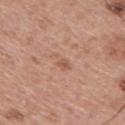Case summary:
* TBP lesion metrics — a footprint of about 3.5 mm², a shape eccentricity near 0.9, and two-axis asymmetry of about 0.4; a border-irregularity index near 4.5/10, a color-variation rating of about 1.5/10, and a peripheral color-asymmetry measure near 0.5; a classifier nevus-likeness of about 0/100 and lesion-presence confidence of about 100/100
* acquisition — ~15 mm tile from a whole-body skin photo
* site — the upper back
* subject — male, about 65 years old
* lighting — white-light